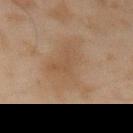Q: Automated lesion metrics?
A: a lesion–skin lightness drop of about 5 and a normalized lesion–skin contrast near 4.5; an automated nevus-likeness rating near 0 out of 100 and a lesion-detection confidence of about 100/100
Q: What is the imaging modality?
A: ~15 mm tile from a whole-body skin photo
Q: How was the tile lit?
A: cross-polarized illumination
Q: Lesion location?
A: the arm
Q: Who is the patient?
A: male, in their mid-40s